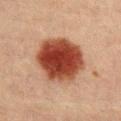The lesion is located on the abdomen.
Cropped from a whole-body photographic skin survey; the tile spans about 15 mm.
Imaged with cross-polarized lighting.
About 6 mm across.
A female subject, aged 38 to 42.